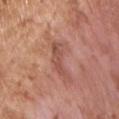<case>
<image>
  <source>total-body photography crop</source>
  <field_of_view_mm>15</field_of_view_mm>
</image>
<patient>
  <sex>male</sex>
  <age_approx>65</age_approx>
</patient>
<site>chest</site>
<automated_metrics>
  <cielab_L>52</cielab_L>
  <cielab_a>24</cielab_a>
  <cielab_b>28</cielab_b>
  <vs_skin_darker_L>8.0</vs_skin_darker_L>
  <vs_skin_contrast_norm>6.0</vs_skin_contrast_norm>
  <peripheral_color_asymmetry>0.5</peripheral_color_asymmetry>
  <nevus_likeness_0_100>0</nevus_likeness_0_100>
</automated_metrics>
</case>